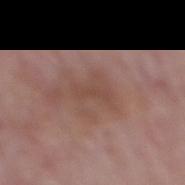<record>
<biopsy_status>not biopsied; imaged during a skin examination</biopsy_status>
<automated_metrics>
  <area_mm2_approx>2.5</area_mm2_approx>
  <shape_asymmetry>0.4</shape_asymmetry>
  <color_variation_0_10>0.0</color_variation_0_10>
  <nevus_likeness_0_100>0</nevus_likeness_0_100>
</automated_metrics>
<lighting>white-light</lighting>
<patient>
  <sex>male</sex>
  <age_approx>65</age_approx>
</patient>
<image>
  <source>total-body photography crop</source>
  <field_of_view_mm>15</field_of_view_mm>
</image>
<site>mid back</site>
</record>